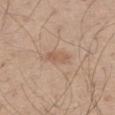Q: Was this lesion biopsied?
A: no biopsy performed (imaged during a skin exam)
Q: What lighting was used for the tile?
A: white-light
Q: Patient demographics?
A: male, aged around 60
Q: Lesion location?
A: the left thigh
Q: What kind of image is this?
A: ~15 mm tile from a whole-body skin photo